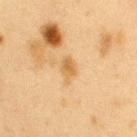<case>
<biopsy_status>not biopsied; imaged during a skin examination</biopsy_status>
<patient>
  <sex>female</sex>
  <age_approx>40</age_approx>
</patient>
<site>left upper arm</site>
<lesion_size>
  <long_diameter_mm_approx>2.5</long_diameter_mm_approx>
</lesion_size>
<image>
  <source>total-body photography crop</source>
  <field_of_view_mm>15</field_of_view_mm>
</image>
<lighting>cross-polarized</lighting>
<automated_metrics>
  <area_mm2_approx>4.0</area_mm2_approx>
  <eccentricity>0.65</eccentricity>
  <shape_asymmetry>0.2</shape_asymmetry>
  <nevus_likeness_0_100>20</nevus_likeness_0_100>
  <lesion_detection_confidence_0_100>100</lesion_detection_confidence_0_100>
</automated_metrics>
</case>